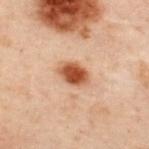Part of a total-body skin-imaging series; this lesion was reviewed on a skin check and was not flagged for biopsy.
A close-up tile cropped from a whole-body skin photograph, about 15 mm across.
The lesion-visualizer software estimated roughly 14 lightness units darker than nearby skin and a normalized border contrast of about 11.5. The analysis additionally found a lesion-detection confidence of about 100/100.
From the upper back.
The patient is a male aged approximately 50.
The recorded lesion diameter is about 3.5 mm.